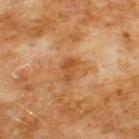Captured during whole-body skin photography for melanoma surveillance; the lesion was not biopsied. Longest diameter approximately 3 mm. On the chest. Imaged with cross-polarized lighting. A male subject roughly 60 years of age. An algorithmic analysis of the crop reported a footprint of about 4.5 mm² and an eccentricity of roughly 0.8. The analysis additionally found a lesion color around L≈50 a*≈25 b*≈40 in CIELAB, a lesion–skin lightness drop of about 9, and a lesion-to-skin contrast of about 6.5 (normalized; higher = more distinct). And it measured border irregularity of about 3.5 on a 0–10 scale, internal color variation of about 3 on a 0–10 scale, and peripheral color asymmetry of about 1. A 15 mm close-up tile from a total-body photography series done for melanoma screening.